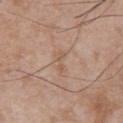biopsy status: no biopsy performed (imaged during a skin exam)
imaging modality: total-body-photography crop, ~15 mm field of view
body site: the chest
subject: male, roughly 50 years of age